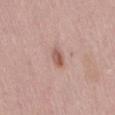| field | value |
|---|---|
| notes | imaged on a skin check; not biopsied |
| subject | female, about 40 years old |
| lesion diameter | ≈2.5 mm |
| location | the lower back |
| imaging modality | ~15 mm crop, total-body skin-cancer survey |
| automated lesion analysis | a lesion color around L≈56 a*≈21 b*≈26 in CIELAB and about 11 CIELAB-L* units darker than the surrounding skin; a border-irregularity rating of about 2/10, internal color variation of about 3.5 on a 0–10 scale, and radial color variation of about 1.5 |
| lighting | white-light |